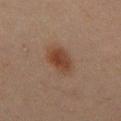patient:
  sex: male
  age_approx: 45
image:
  source: total-body photography crop
  field_of_view_mm: 15
site: mid back